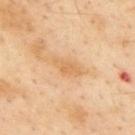The recorded lesion diameter is about 4 mm. The lesion is on the back. Cropped from a total-body skin-imaging series; the visible field is about 15 mm. The lesion-visualizer software estimated a footprint of about 5 mm², an eccentricity of roughly 0.9, and two-axis asymmetry of about 0.35. A male subject about 55 years old. The tile uses cross-polarized illumination.